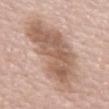Captured during whole-body skin photography for melanoma surveillance; the lesion was not biopsied. The lesion is located on the back. Captured under white-light illumination. Cropped from a total-body skin-imaging series; the visible field is about 15 mm. A male subject, roughly 75 years of age.